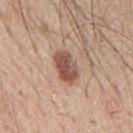follow-up=imaged on a skin check; not biopsied | acquisition=15 mm crop, total-body photography | lighting=white-light | subject=male, approximately 65 years of age | location=the mid back.